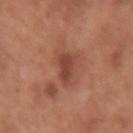Imaged during a routine full-body skin examination; the lesion was not biopsied and no histopathology is available.
The total-body-photography lesion software estimated a nevus-likeness score of about 5/100 and a lesion-detection confidence of about 100/100.
On the right upper arm.
About 3 mm across.
This is a white-light tile.
A female patient, aged approximately 50.
A 15 mm close-up tile from a total-body photography series done for melanoma screening.